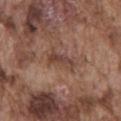The lesion was tiled from a total-body skin photograph and was not biopsied.